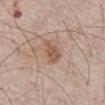Notes:
- follow-up: no biopsy performed (imaged during a skin exam)
- tile lighting: white-light
- image-analysis metrics: an average lesion color of about L≈55 a*≈19 b*≈29 (CIELAB), about 9 CIELAB-L* units darker than the surrounding skin, and a normalized border contrast of about 7; border irregularity of about 3 on a 0–10 scale and a within-lesion color-variation index near 1.5/10; a lesion-detection confidence of about 100/100
- subject: male, aged around 65
- lesion size: about 4 mm
- image: ~15 mm crop, total-body skin-cancer survey
- location: the chest